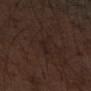No biopsy was performed on this lesion — it was imaged during a full skin examination and was not determined to be concerning.
Approximately 3.5 mm at its widest.
Located on the left forearm.
A close-up tile cropped from a whole-body skin photograph, about 15 mm across.
A male patient, approximately 55 years of age.